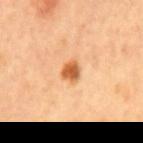This lesion was catalogued during total-body skin photography and was not selected for biopsy. A 15 mm crop from a total-body photograph taken for skin-cancer surveillance. The patient is a male in their mid- to late 60s. The recorded lesion diameter is about 2.5 mm. Located on the mid back.